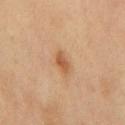Clinical impression: Recorded during total-body skin imaging; not selected for excision or biopsy. Background: Measured at roughly 3 mm in maximum diameter. A 15 mm close-up extracted from a 3D total-body photography capture. The lesion is located on the chest. The subject is a male in their mid-60s.